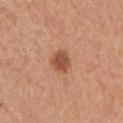The lesion was tiled from a total-body skin photograph and was not biopsied.
The recorded lesion diameter is about 3 mm.
This is a white-light tile.
The subject is a female aged 43–47.
On the left upper arm.
A close-up tile cropped from a whole-body skin photograph, about 15 mm across.
An algorithmic analysis of the crop reported a detector confidence of about 100 out of 100 that the crop contains a lesion.